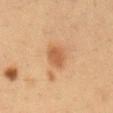Clinical impression:
This lesion was catalogued during total-body skin photography and was not selected for biopsy.
Image and clinical context:
The subject is a male aged 33–37. From the mid back. The recorded lesion diameter is about 3 mm. Cropped from a whole-body photographic skin survey; the tile spans about 15 mm. Captured under cross-polarized illumination. An algorithmic analysis of the crop reported a lesion area of about 5.5 mm² and an outline eccentricity of about 0.55 (0 = round, 1 = elongated). It also reported an average lesion color of about L≈53 a*≈22 b*≈36 (CIELAB) and about 10 CIELAB-L* units darker than the surrounding skin. The software also gave border irregularity of about 2 on a 0–10 scale, internal color variation of about 2 on a 0–10 scale, and peripheral color asymmetry of about 0.5.